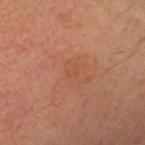The lesion was photographed on a routine skin check and not biopsied; there is no pathology result. The lesion-visualizer software estimated a footprint of about 1.5 mm², a shape eccentricity near 0.75, and a symmetry-axis asymmetry near 0.3. And it measured a lesion color around L≈50 a*≈27 b*≈35 in CIELAB, a lesion–skin lightness drop of about 3, and a normalized lesion–skin contrast near 3.5. The software also gave an automated nevus-likeness rating near 0 out of 100. This is a cross-polarized tile. Measured at roughly 1.5 mm in maximum diameter. A male patient aged approximately 50. Located on the head or neck. A 15 mm crop from a total-body photograph taken for skin-cancer surveillance.